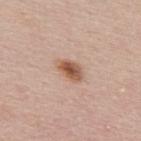No biopsy was performed on this lesion — it was imaged during a full skin examination and was not determined to be concerning. A male subject approximately 50 years of age. A lesion tile, about 15 mm wide, cut from a 3D total-body photograph. From the upper back. About 3.5 mm across. Automated image analysis of the tile measured a footprint of about 5.5 mm², a shape eccentricity near 0.8, and a shape-asymmetry score of about 0.2 (0 = symmetric). The analysis additionally found a border-irregularity rating of about 1.5/10 and a color-variation rating of about 5.5/10. It also reported lesion-presence confidence of about 100/100.